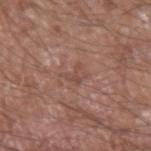Impression:
The lesion was tiled from a total-body skin photograph and was not biopsied.
Context:
Measured at roughly 3 mm in maximum diameter. From the left upper arm. A male patient, roughly 65 years of age. The lesion-visualizer software estimated an eccentricity of roughly 0.75. It also reported a classifier nevus-likeness of about 0/100 and a detector confidence of about 95 out of 100 that the crop contains a lesion. A roughly 15 mm field-of-view crop from a total-body skin photograph. Imaged with white-light lighting.